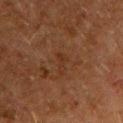Part of a total-body skin-imaging series; this lesion was reviewed on a skin check and was not flagged for biopsy.
Located on the chest.
A male subject aged around 60.
Approximately 2.5 mm at its widest.
The lesion-visualizer software estimated an outline eccentricity of about 0.95 (0 = round, 1 = elongated). The software also gave a mean CIELAB color near L≈24 a*≈17 b*≈25, about 4 CIELAB-L* units darker than the surrounding skin, and a normalized lesion–skin contrast near 5. It also reported a border-irregularity rating of about 5.5/10, internal color variation of about 0 on a 0–10 scale, and peripheral color asymmetry of about 0. It also reported lesion-presence confidence of about 100/100.
A 15 mm crop from a total-body photograph taken for skin-cancer surveillance.